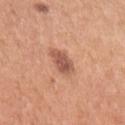notes = no biopsy performed (imaged during a skin exam) | diameter = about 3.5 mm | lighting = white-light illumination | acquisition = 15 mm crop, total-body photography | body site = the arm | patient = female, about 30 years old.